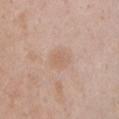Notes:
• biopsy status · total-body-photography surveillance lesion; no biopsy
• imaging modality · 15 mm crop, total-body photography
• site · the chest
• patient · female, aged around 40